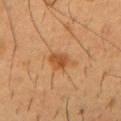follow-up=catalogued during a skin exam; not biopsied
body site=the chest
acquisition=~15 mm tile from a whole-body skin photo
subject=male, approximately 55 years of age
lesion size=~3.5 mm (longest diameter)
image-analysis metrics=an average lesion color of about L≈44 a*≈22 b*≈36 (CIELAB) and a lesion–skin lightness drop of about 9
illumination=cross-polarized illumination